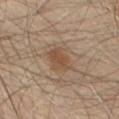<tbp_lesion>
<biopsy_status>not biopsied; imaged during a skin examination</biopsy_status>
<patient>
  <sex>male</sex>
  <age_approx>70</age_approx>
</patient>
<image>
  <source>total-body photography crop</source>
  <field_of_view_mm>15</field_of_view_mm>
</image>
<automated_metrics>
  <area_mm2_approx>6.5</area_mm2_approx>
  <eccentricity>0.7</eccentricity>
  <shape_asymmetry>0.25</shape_asymmetry>
  <cielab_L>47</cielab_L>
  <cielab_a>17</cielab_a>
  <cielab_b>29</cielab_b>
  <vs_skin_darker_L>8.0</vs_skin_darker_L>
  <vs_skin_contrast_norm>6.5</vs_skin_contrast_norm>
  <lesion_detection_confidence_0_100>100</lesion_detection_confidence_0_100>
</automated_metrics>
<site>abdomen</site>
<lesion_size>
  <long_diameter_mm_approx>3.0</long_diameter_mm_approx>
</lesion_size>
</tbp_lesion>